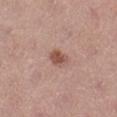Clinical impression:
Recorded during total-body skin imaging; not selected for excision or biopsy.
Acquisition and patient details:
A lesion tile, about 15 mm wide, cut from a 3D total-body photograph. The recorded lesion diameter is about 2.5 mm. Automated image analysis of the tile measured a shape eccentricity near 0.7 and a symmetry-axis asymmetry near 0.2. And it measured a lesion color around L≈52 a*≈22 b*≈26 in CIELAB, a lesion–skin lightness drop of about 11, and a lesion-to-skin contrast of about 8 (normalized; higher = more distinct). It also reported border irregularity of about 1.5 on a 0–10 scale, a within-lesion color-variation index near 2/10, and peripheral color asymmetry of about 0.5. The software also gave a nevus-likeness score of about 90/100 and a detector confidence of about 100 out of 100 that the crop contains a lesion. The lesion is on the right lower leg. A female patient approximately 45 years of age. Imaged with white-light lighting.